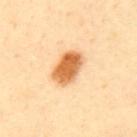Assessment:
Captured during whole-body skin photography for melanoma surveillance; the lesion was not biopsied.